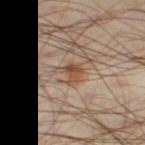The lesion was photographed on a routine skin check and not biopsied; there is no pathology result.
Measured at roughly 3 mm in maximum diameter.
A male subject, about 40 years old.
A close-up tile cropped from a whole-body skin photograph, about 15 mm across.
The tile uses cross-polarized illumination.
The lesion is on the right thigh.
Automated image analysis of the tile measured a shape eccentricity near 0.5 and a shape-asymmetry score of about 0.45 (0 = symmetric). And it measured a border-irregularity index near 4.5/10 and a color-variation rating of about 5.5/10.